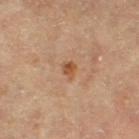follow-up = catalogued during a skin exam; not biopsied
image = ~15 mm tile from a whole-body skin photo
patient = female, aged approximately 65
illumination = cross-polarized illumination
site = the right thigh
TBP lesion metrics = a lesion area of about 2.5 mm² and a shape eccentricity near 0.6; radial color variation of about 1; a lesion-detection confidence of about 100/100